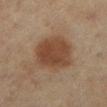workup: total-body-photography surveillance lesion; no biopsy | anatomic site: the left lower leg | image: total-body-photography crop, ~15 mm field of view | subject: female, aged around 55 | automated lesion analysis: an eccentricity of roughly 0.65 and a shape-asymmetry score of about 0.2 (0 = symmetric); a classifier nevus-likeness of about 100/100 and lesion-presence confidence of about 100/100 | size: about 6 mm | lighting: cross-polarized.